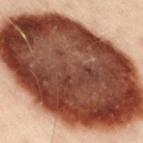Q: Is there a histopathology result?
A: no biopsy performed (imaged during a skin exam)
Q: Patient demographics?
A: male, about 50 years old
Q: What is the imaging modality?
A: ~15 mm tile from a whole-body skin photo
Q: Lesion location?
A: the lower back
Q: How was the tile lit?
A: cross-polarized
Q: How large is the lesion?
A: about 17 mm